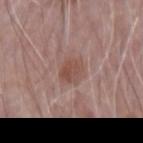workup: catalogued during a skin exam; not biopsied
patient: male, in their 70s
lesion diameter: ~3 mm (longest diameter)
image: ~15 mm tile from a whole-body skin photo
TBP lesion metrics: an area of roughly 5 mm², a shape eccentricity near 0.75, and a shape-asymmetry score of about 0.2 (0 = symmetric); a lesion color around L≈49 a*≈20 b*≈25 in CIELAB, about 8 CIELAB-L* units darker than the surrounding skin, and a normalized lesion–skin contrast near 6.5; a color-variation rating of about 3/10
body site: the right forearm